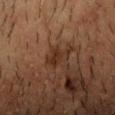follow-up: total-body-photography surveillance lesion; no biopsy | site: the head or neck | subject: male, aged approximately 50 | diameter: about 3 mm | acquisition: ~15 mm crop, total-body skin-cancer survey.